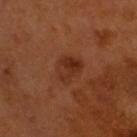  biopsy_status: not biopsied; imaged during a skin examination
  lighting: cross-polarized
  patient:
    sex: male
    age_approx: 60
  image:
    source: total-body photography crop
    field_of_view_mm: 15
  lesion_size:
    long_diameter_mm_approx: 4.0
  site: head or neck